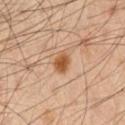Recorded during total-body skin imaging; not selected for excision or biopsy.
The lesion is located on the chest.
Imaged with cross-polarized lighting.
A 15 mm close-up tile from a total-body photography series done for melanoma screening.
The lesion's longest dimension is about 2.5 mm.
The patient is a male roughly 35 years of age.
Automated image analysis of the tile measured a lesion area of about 4.5 mm², an outline eccentricity of about 0.65 (0 = round, 1 = elongated), and a shape-asymmetry score of about 0.25 (0 = symmetric). The analysis additionally found a lesion color around L≈52 a*≈22 b*≈36 in CIELAB, roughly 12 lightness units darker than nearby skin, and a lesion-to-skin contrast of about 9.5 (normalized; higher = more distinct). And it measured an automated nevus-likeness rating near 100 out of 100 and a lesion-detection confidence of about 100/100.